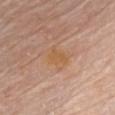The lesion was photographed on a routine skin check and not biopsied; there is no pathology result. The subject is a male aged approximately 75. A 15 mm close-up tile from a total-body photography series done for melanoma screening. Longest diameter approximately 2.5 mm. Located on the chest.